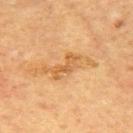Impression:
Recorded during total-body skin imaging; not selected for excision or biopsy.
Clinical summary:
A male subject in their mid- to late 60s. The lesion-visualizer software estimated an area of roughly 5 mm², an outline eccentricity of about 0.9 (0 = round, 1 = elongated), and two-axis asymmetry of about 0.5. It also reported a lesion color around L≈55 a*≈21 b*≈41 in CIELAB, roughly 9 lightness units darker than nearby skin, and a normalized border contrast of about 6.5. From the back. This is a cross-polarized tile. A 15 mm close-up extracted from a 3D total-body photography capture.